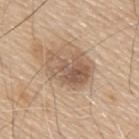Notes:
• body site · the upper back
• image-analysis metrics · a border-irregularity index near 4/10 and a within-lesion color-variation index near 7/10; a nevus-likeness score of about 25/100
• illumination · white-light illumination
• diameter · ~5 mm (longest diameter)
• image · ~15 mm crop, total-body skin-cancer survey
• patient · male, in their 80s